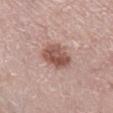Case summary:
* follow-up · no biopsy performed (imaged during a skin exam)
* image source · 15 mm crop, total-body photography
* patient · female, aged 63–67
* diameter · ≈3.5 mm
* image-analysis metrics · radial color variation of about 2; a classifier nevus-likeness of about 85/100 and lesion-presence confidence of about 100/100
* location · the right lower leg
* illumination · white-light